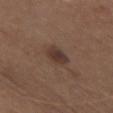Q: Who is the patient?
A: female, aged 48 to 52
Q: What kind of image is this?
A: ~15 mm tile from a whole-body skin photo
Q: Lesion size?
A: ~3.5 mm (longest diameter)
Q: Where on the body is the lesion?
A: the front of the torso
Q: What did automated image analysis measure?
A: a lesion color around L≈36 a*≈16 b*≈22 in CIELAB, about 9 CIELAB-L* units darker than the surrounding skin, and a lesion-to-skin contrast of about 8 (normalized; higher = more distinct)
Q: What lighting was used for the tile?
A: white-light